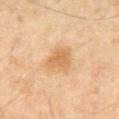Part of a total-body skin-imaging series; this lesion was reviewed on a skin check and was not flagged for biopsy.
The lesion is located on the front of the torso.
Imaged with cross-polarized lighting.
The lesion's longest dimension is about 3.5 mm.
A close-up tile cropped from a whole-body skin photograph, about 15 mm across.
A male patient roughly 65 years of age.